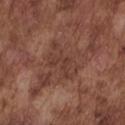Captured during whole-body skin photography for melanoma surveillance; the lesion was not biopsied. A lesion tile, about 15 mm wide, cut from a 3D total-body photograph. The lesion's longest dimension is about 5 mm. The lesion is on the chest. The tile uses white-light illumination. The subject is a male roughly 75 years of age.